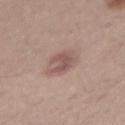The lesion was tiled from a total-body skin photograph and was not biopsied.
A male patient, roughly 30 years of age.
Located on the arm.
A 15 mm close-up tile from a total-body photography series done for melanoma screening.